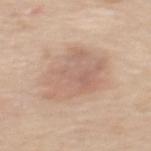  lesion_size:
    long_diameter_mm_approx: 6.5
  image:
    source: total-body photography crop
    field_of_view_mm: 15
  lighting: white-light
  patient:
    sex: female
    age_approx: 65
  site: upper back